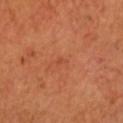Q: Was a biopsy performed?
A: catalogued during a skin exam; not biopsied